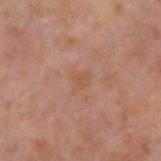The lesion was tiled from a total-body skin photograph and was not biopsied.
The lesion's longest dimension is about 2.5 mm.
This is a cross-polarized tile.
The lesion is located on the left forearm.
Cropped from a whole-body photographic skin survey; the tile spans about 15 mm.
A male patient, approximately 30 years of age.